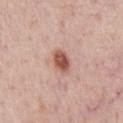Clinical impression:
This lesion was catalogued during total-body skin photography and was not selected for biopsy.
Context:
Longest diameter approximately 3 mm. A male patient, aged around 60. From the chest. The tile uses white-light illumination. This image is a 15 mm lesion crop taken from a total-body photograph.